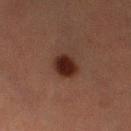• workup · imaged on a skin check; not biopsied
• subject · female, in their mid-50s
• lesion diameter · ~3 mm (longest diameter)
• imaging modality · 15 mm crop, total-body photography
• body site · the left thigh
• lighting · cross-polarized illumination